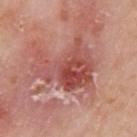- workup: catalogued during a skin exam; not biopsied
- diameter: ≈7.5 mm
- subject: male, approximately 75 years of age
- location: the left upper arm
- imaging modality: ~15 mm tile from a whole-body skin photo